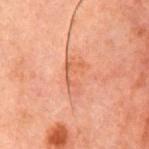Part of a total-body skin-imaging series; this lesion was reviewed on a skin check and was not flagged for biopsy. A 15 mm close-up extracted from a 3D total-body photography capture. A male subject aged 58 to 62. Measured at roughly 4 mm in maximum diameter. From the chest. This is a cross-polarized tile.